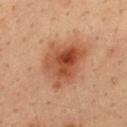Impression: Captured during whole-body skin photography for melanoma surveillance; the lesion was not biopsied. Image and clinical context: Automated tile analysis of the lesion measured border irregularity of about 3 on a 0–10 scale and a within-lesion color-variation index near 8/10. It also reported a nevus-likeness score of about 95/100. The subject is a male approximately 35 years of age. Measured at roughly 6 mm in maximum diameter. A roughly 15 mm field-of-view crop from a total-body skin photograph. The lesion is located on the upper back. Captured under cross-polarized illumination.